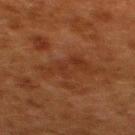<tbp_lesion>
<biopsy_status>not biopsied; imaged during a skin examination</biopsy_status>
<lighting>cross-polarized</lighting>
<patient>
  <sex>female</sex>
  <age_approx>50</age_approx>
</patient>
<image>
  <source>total-body photography crop</source>
  <field_of_view_mm>15</field_of_view_mm>
</image>
<site>upper back</site>
</tbp_lesion>